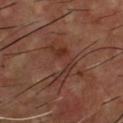Clinical impression:
The lesion was tiled from a total-body skin photograph and was not biopsied.
Image and clinical context:
Located on the chest. A 15 mm close-up extracted from a 3D total-body photography capture. The patient is a male aged 53–57.